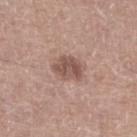Impression: The lesion was tiled from a total-body skin photograph and was not biopsied. Background: A region of skin cropped from a whole-body photographic capture, roughly 15 mm wide. A male subject, approximately 65 years of age. Located on the left lower leg.